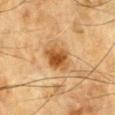Captured during whole-body skin photography for melanoma surveillance; the lesion was not biopsied. A male patient aged 83 or older. This image is a 15 mm lesion crop taken from a total-body photograph. Captured under cross-polarized illumination. On the chest.